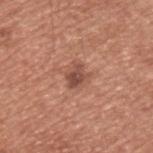{"biopsy_status": "not biopsied; imaged during a skin examination", "patient": {"sex": "male", "age_approx": 55}, "lighting": "white-light", "image": {"source": "total-body photography crop", "field_of_view_mm": 15}, "automated_metrics": {"border_irregularity_0_10": 3.0, "color_variation_0_10": 4.0, "peripheral_color_asymmetry": 1.5}, "lesion_size": {"long_diameter_mm_approx": 3.0}, "site": "upper back"}